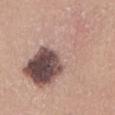This lesion was catalogued during total-body skin photography and was not selected for biopsy. About 11 mm across. This is a white-light tile. The total-body-photography lesion software estimated a lesion–skin lightness drop of about 12 and a normalized lesion–skin contrast near 8. And it measured a border-irregularity index near 8.5/10, internal color variation of about 10 on a 0–10 scale, and radial color variation of about 7. The software also gave a classifier nevus-likeness of about 5/100 and lesion-presence confidence of about 100/100. Located on the front of the torso. The subject is a female roughly 35 years of age. A close-up tile cropped from a whole-body skin photograph, about 15 mm across.